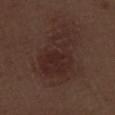| field | value |
|---|---|
| workup | imaged on a skin check; not biopsied |
| diameter | about 6.5 mm |
| location | the left thigh |
| subject | male, in their 70s |
| image source | 15 mm crop, total-body photography |